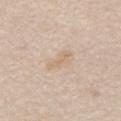<case>
<biopsy_status>not biopsied; imaged during a skin examination</biopsy_status>
<automated_metrics>
  <area_mm2_approx>3.5</area_mm2_approx>
  <eccentricity>0.95</eccentricity>
  <shape_asymmetry>0.35</shape_asymmetry>
  <cielab_L>68</cielab_L>
  <cielab_a>14</cielab_a>
  <cielab_b>31</cielab_b>
  <vs_skin_darker_L>6.0</vs_skin_darker_L>
  <vs_skin_contrast_norm>5.0</vs_skin_contrast_norm>
  <lesion_detection_confidence_0_100>90</lesion_detection_confidence_0_100>
</automated_metrics>
<patient>
  <sex>male</sex>
  <age_approx>65</age_approx>
</patient>
<image>
  <source>total-body photography crop</source>
  <field_of_view_mm>15</field_of_view_mm>
</image>
<site>mid back</site>
<lighting>white-light</lighting>
</case>